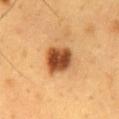<lesion>
  <biopsy_status>not biopsied; imaged during a skin examination</biopsy_status>
  <site>mid back</site>
  <lighting>cross-polarized</lighting>
  <lesion_size>
    <long_diameter_mm_approx>3.5</long_diameter_mm_approx>
  </lesion_size>
  <patient>
    <sex>male</sex>
    <age_approx>60</age_approx>
  </patient>
  <image>
    <source>total-body photography crop</source>
    <field_of_view_mm>15</field_of_view_mm>
  </image>
</lesion>